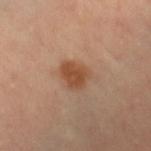The lesion was tiled from a total-body skin photograph and was not biopsied. A female patient in their 40s. A lesion tile, about 15 mm wide, cut from a 3D total-body photograph. Automated image analysis of the tile measured a lesion area of about 7 mm² and an outline eccentricity of about 0.65 (0 = round, 1 = elongated). It also reported a mean CIELAB color near L≈49 a*≈23 b*≈34, roughly 10 lightness units darker than nearby skin, and a normalized lesion–skin contrast near 8.5. Measured at roughly 3.5 mm in maximum diameter. Imaged with cross-polarized lighting. On the left leg.